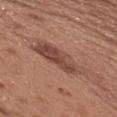workup: catalogued during a skin exam; not biopsied | diameter: about 6.5 mm | acquisition: ~15 mm tile from a whole-body skin photo | patient: male, in their mid-50s | body site: the chest | illumination: white-light.